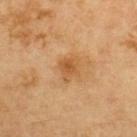biopsy status = no biopsy performed (imaged during a skin exam)
site = the back
patient = male, in their 70s
image = ~15 mm tile from a whole-body skin photo